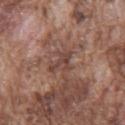The lesion was photographed on a routine skin check and not biopsied; there is no pathology result.